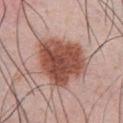Q: Is there a histopathology result?
A: catalogued during a skin exam; not biopsied
Q: What are the patient's age and sex?
A: male, aged 33–37
Q: How was the tile lit?
A: white-light illumination
Q: Lesion location?
A: the front of the torso
Q: What kind of image is this?
A: ~15 mm crop, total-body skin-cancer survey